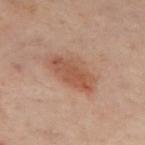automated metrics=an eccentricity of roughly 0.85 and two-axis asymmetry of about 0.2; a border-irregularity index near 2/10, a color-variation rating of about 3.5/10, and radial color variation of about 1.5 | subject=male, roughly 50 years of age | tile lighting=cross-polarized | size=about 5.5 mm | acquisition=~15 mm crop, total-body skin-cancer survey | anatomic site=the mid back.